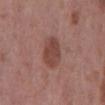Recorded during total-body skin imaging; not selected for excision or biopsy. A 15 mm crop from a total-body photograph taken for skin-cancer surveillance. This is a white-light tile. The lesion is located on the mid back. Automated tile analysis of the lesion measured an eccentricity of roughly 0.6 and a symmetry-axis asymmetry near 0.1. It also reported a lesion color around L≈44 a*≈22 b*≈23 in CIELAB and a normalized border contrast of about 7.5. The software also gave a border-irregularity rating of about 1/10, a within-lesion color-variation index near 2/10, and radial color variation of about 0.5. The software also gave a nevus-likeness score of about 60/100 and lesion-presence confidence of about 100/100. The subject is a male in their 60s. Approximately 4 mm at its widest.